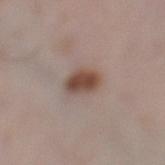Q: Is there a histopathology result?
A: imaged on a skin check; not biopsied
Q: How was this image acquired?
A: total-body-photography crop, ~15 mm field of view
Q: Patient demographics?
A: female, roughly 30 years of age
Q: What is the anatomic site?
A: the left lower leg
Q: What is the lesion's diameter?
A: about 4 mm